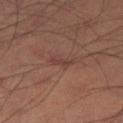Clinical impression: The lesion was tiled from a total-body skin photograph and was not biopsied. Background: A 15 mm close-up extracted from a 3D total-body photography capture. On the leg. A male patient about 50 years old. Imaged with cross-polarized lighting. The lesion's longest dimension is about 3 mm.